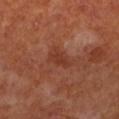workup = total-body-photography surveillance lesion; no biopsy | patient = male, approximately 70 years of age | anatomic site = the left lower leg | imaging modality = 15 mm crop, total-body photography.